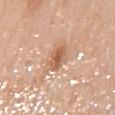On the mid back. Automated tile analysis of the lesion measured a footprint of about 5.5 mm². The software also gave border irregularity of about 2.5 on a 0–10 scale and a peripheral color-asymmetry measure near 1. The patient is a female about 50 years old. A 15 mm crop from a total-body photograph taken for skin-cancer surveillance.